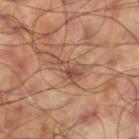workup = imaged on a skin check; not biopsied | body site = the left thigh | size = about 4 mm | patient = male, roughly 60 years of age | acquisition = ~15 mm crop, total-body skin-cancer survey | illumination = cross-polarized illumination.